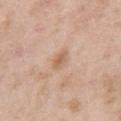The lesion was tiled from a total-body skin photograph and was not biopsied.
Located on the left upper arm.
A male patient in their 50s.
Cropped from a whole-body photographic skin survey; the tile spans about 15 mm.
The tile uses white-light illumination.